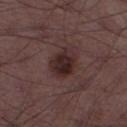Recorded during total-body skin imaging; not selected for excision or biopsy. A male patient, aged 48 to 52. A 15 mm close-up tile from a total-body photography series done for melanoma screening. About 3.5 mm across. The tile uses white-light illumination. The total-body-photography lesion software estimated a mean CIELAB color near L≈25 a*≈17 b*≈16, roughly 10 lightness units darker than nearby skin, and a lesion-to-skin contrast of about 10.5 (normalized; higher = more distinct). The analysis additionally found border irregularity of about 2 on a 0–10 scale, a within-lesion color-variation index near 3.5/10, and a peripheral color-asymmetry measure near 1. On the left thigh.